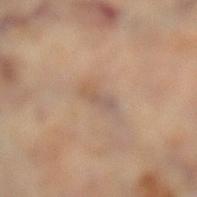Captured during whole-body skin photography for melanoma surveillance; the lesion was not biopsied. The lesion is on the leg. The subject is a female roughly 60 years of age. A roughly 15 mm field-of-view crop from a total-body skin photograph.